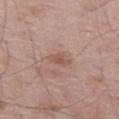Measured at roughly 3 mm in maximum diameter. A lesion tile, about 15 mm wide, cut from a 3D total-body photograph. A male subject, in their 50s. This is a white-light tile. From the leg. An algorithmic analysis of the crop reported an average lesion color of about L≈54 a*≈21 b*≈25 (CIELAB), a lesion–skin lightness drop of about 8, and a lesion-to-skin contrast of about 6 (normalized; higher = more distinct). It also reported a detector confidence of about 100 out of 100 that the crop contains a lesion.